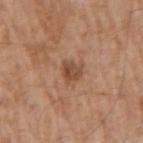{
  "biopsy_status": "not biopsied; imaged during a skin examination",
  "image": {
    "source": "total-body photography crop",
    "field_of_view_mm": 15
  },
  "site": "right upper arm",
  "patient": {
    "sex": "male",
    "age_approx": 65
  }
}